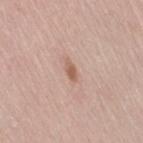Part of a total-body skin-imaging series; this lesion was reviewed on a skin check and was not flagged for biopsy.
Approximately 2.5 mm at its widest.
A close-up tile cropped from a whole-body skin photograph, about 15 mm across.
The tile uses white-light illumination.
An algorithmic analysis of the crop reported a mean CIELAB color near L≈59 a*≈20 b*≈28, roughly 10 lightness units darker than nearby skin, and a normalized lesion–skin contrast near 7. The software also gave border irregularity of about 3 on a 0–10 scale, internal color variation of about 1 on a 0–10 scale, and peripheral color asymmetry of about 0. The analysis additionally found a classifier nevus-likeness of about 75/100 and a detector confidence of about 100 out of 100 that the crop contains a lesion.
A female subject, roughly 65 years of age.
The lesion is on the right thigh.